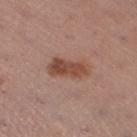Recorded during total-body skin imaging; not selected for excision or biopsy. About 4.5 mm across. The lesion is located on the left lower leg. A 15 mm close-up extracted from a 3D total-body photography capture. A female subject, aged around 55. Automated tile analysis of the lesion measured a lesion area of about 8.5 mm², an outline eccentricity of about 0.9 (0 = round, 1 = elongated), and a shape-asymmetry score of about 0.2 (0 = symmetric). The software also gave a border-irregularity index near 2.5/10 and radial color variation of about 1.5.